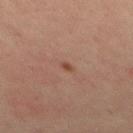– notes: catalogued during a skin exam; not biopsied
– subject: male, in their 30s
– size: about 1 mm
– anatomic site: the mid back
– image: total-body-photography crop, ~15 mm field of view